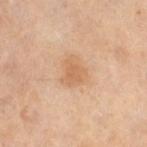  patient:
    sex: female
    age_approx: 50
  site: leg
  image:
    source: total-body photography crop
    field_of_view_mm: 15
  lighting: cross-polarized